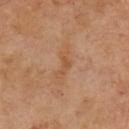Assessment: No biopsy was performed on this lesion — it was imaged during a full skin examination and was not determined to be concerning. Clinical summary: The tile uses cross-polarized illumination. Automated tile analysis of the lesion measured a classifier nevus-likeness of about 0/100 and lesion-presence confidence of about 100/100. Approximately 4 mm at its widest. A 15 mm crop from a total-body photograph taken for skin-cancer surveillance. The patient is a male roughly 65 years of age.